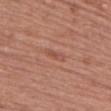  biopsy_status: not biopsied; imaged during a skin examination
  lesion_size:
    long_diameter_mm_approx: 3.0
  lighting: white-light
  site: right upper arm
  image:
    source: total-body photography crop
    field_of_view_mm: 15
  patient:
    sex: female
    age_approx: 65